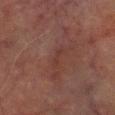{"biopsy_status": "not biopsied; imaged during a skin examination", "lesion_size": {"long_diameter_mm_approx": 2.5}, "image": {"source": "total-body photography crop", "field_of_view_mm": 15}, "automated_metrics": {"cielab_L": 28, "cielab_a": 18, "cielab_b": 20, "vs_skin_darker_L": 4.0, "vs_skin_contrast_norm": 4.5, "lesion_detection_confidence_0_100": 70}, "patient": {"sex": "male", "age_approx": 70}, "site": "left lower leg", "lighting": "cross-polarized"}